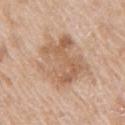| feature | finding |
|---|---|
| biopsy status | imaged on a skin check; not biopsied |
| TBP lesion metrics | a footprint of about 31 mm² and a shape-asymmetry score of about 0.25 (0 = symmetric); a lesion color around L≈62 a*≈18 b*≈32 in CIELAB and about 9 CIELAB-L* units darker than the surrounding skin; a border-irregularity index near 3.5/10, a color-variation rating of about 5.5/10, and peripheral color asymmetry of about 1.5 |
| illumination | white-light illumination |
| site | the arm |
| acquisition | total-body-photography crop, ~15 mm field of view |
| subject | male, approximately 65 years of age |
| lesion size | ≈7.5 mm |